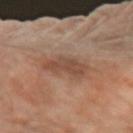Captured during whole-body skin photography for melanoma surveillance; the lesion was not biopsied.
Located on the left forearm.
The lesion's longest dimension is about 4 mm.
A female patient, aged approximately 70.
A roughly 15 mm field-of-view crop from a total-body skin photograph.